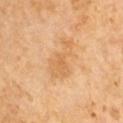Part of a total-body skin-imaging series; this lesion was reviewed on a skin check and was not flagged for biopsy. The total-body-photography lesion software estimated a footprint of about 10 mm², a shape eccentricity near 0.9, and a shape-asymmetry score of about 0.5 (0 = symmetric). Located on the upper back. A roughly 15 mm field-of-view crop from a total-body skin photograph. A male patient aged approximately 60. Imaged with cross-polarized lighting. About 5 mm across.